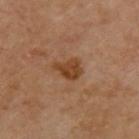lesion size = ~3 mm (longest diameter)
location = the upper back
image = ~15 mm tile from a whole-body skin photo